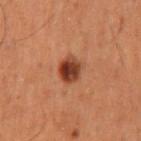notes — no biopsy performed (imaged during a skin exam); patient — male, about 60 years old; lighting — cross-polarized illumination; body site — the lower back; imaging modality — 15 mm crop, total-body photography.